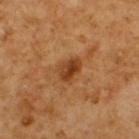<record>
  <biopsy_status>not biopsied; imaged during a skin examination</biopsy_status>
  <lesion_size>
    <long_diameter_mm_approx>3.0</long_diameter_mm_approx>
  </lesion_size>
  <image>
    <source>total-body photography crop</source>
    <field_of_view_mm>15</field_of_view_mm>
  </image>
  <patient>
    <sex>male</sex>
    <age_approx>60</age_approx>
  </patient>
  <lighting>cross-polarized</lighting>
  <site>back</site>
</record>